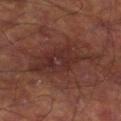- biopsy status — imaged on a skin check; not biopsied
- subject — male, aged 58 to 62
- imaging modality — total-body-photography crop, ~15 mm field of view
- lighting — cross-polarized
- anatomic site — the right lower leg
- lesion diameter — ~9 mm (longest diameter)